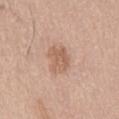Findings:
• biopsy status · total-body-photography surveillance lesion; no biopsy
• patient · male, aged 73–77
• body site · the back
• lesion size · ≈3.5 mm
• imaging modality · 15 mm crop, total-body photography